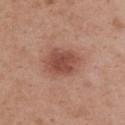Captured during whole-body skin photography for melanoma surveillance; the lesion was not biopsied. Cropped from a whole-body photographic skin survey; the tile spans about 15 mm. On the upper back. An algorithmic analysis of the crop reported a lesion color around L≈49 a*≈24 b*≈27 in CIELAB, about 11 CIELAB-L* units darker than the surrounding skin, and a lesion-to-skin contrast of about 8 (normalized; higher = more distinct). The analysis additionally found a border-irregularity rating of about 1.5/10, a within-lesion color-variation index near 3/10, and a peripheral color-asymmetry measure near 1. The tile uses white-light illumination. The lesion's longest dimension is about 4.5 mm. A female subject approximately 40 years of age.